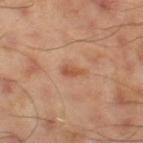Clinical impression: Imaged during a routine full-body skin examination; the lesion was not biopsied and no histopathology is available. Background: Imaged with cross-polarized lighting. The lesion is on the leg. A male patient roughly 45 years of age. This image is a 15 mm lesion crop taken from a total-body photograph.